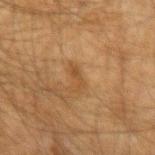The lesion was tiled from a total-body skin photograph and was not biopsied.
The subject is a male aged 48 to 52.
The lesion's longest dimension is about 2.5 mm.
On the right forearm.
The tile uses cross-polarized illumination.
Cropped from a total-body skin-imaging series; the visible field is about 15 mm.